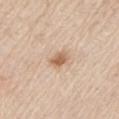Captured during whole-body skin photography for melanoma surveillance; the lesion was not biopsied. The lesion is located on the left upper arm. The patient is a male aged 78 to 82. Imaged with white-light lighting. A close-up tile cropped from a whole-body skin photograph, about 15 mm across.